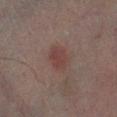This lesion was catalogued during total-body skin photography and was not selected for biopsy. A 15 mm close-up tile from a total-body photography series done for melanoma screening. About 3 mm across. The lesion is on the left lower leg. The subject is a male approximately 70 years of age.